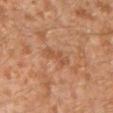| key | value |
|---|---|
| notes | catalogued during a skin exam; not biopsied |
| image source | 15 mm crop, total-body photography |
| subject | male, aged 28 to 32 |
| anatomic site | the left lower leg |
| tile lighting | cross-polarized illumination |
| size | ~4 mm (longest diameter) |